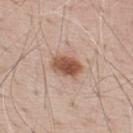patient — male, roughly 70 years of age
lesion diameter — ~3.5 mm (longest diameter)
image source — total-body-photography crop, ~15 mm field of view
illumination — white-light
body site — the upper back